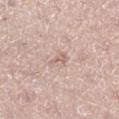Q: Was a biopsy performed?
A: total-body-photography surveillance lesion; no biopsy
Q: What kind of image is this?
A: ~15 mm crop, total-body skin-cancer survey
Q: What did automated image analysis measure?
A: a footprint of about 2 mm² and an outline eccentricity of about 0.9 (0 = round, 1 = elongated); roughly 9 lightness units darker than nearby skin and a normalized border contrast of about 5.5
Q: What is the anatomic site?
A: the right lower leg
Q: Who is the patient?
A: female, approximately 35 years of age
Q: How was the tile lit?
A: white-light
Q: Lesion size?
A: ≈2.5 mm